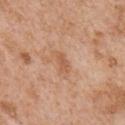anatomic site=the chest; size=≈2.5 mm; tile lighting=white-light; image=~15 mm tile from a whole-body skin photo; patient=male, roughly 65 years of age.